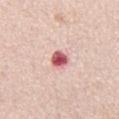Impression: Imaged during a routine full-body skin examination; the lesion was not biopsied and no histopathology is available. Acquisition and patient details: A region of skin cropped from a whole-body photographic capture, roughly 15 mm wide. Longest diameter approximately 2.5 mm. An algorithmic analysis of the crop reported a lesion color around L≈59 a*≈32 b*≈22 in CIELAB, about 19 CIELAB-L* units darker than the surrounding skin, and a normalized border contrast of about 11.5. The analysis additionally found a border-irregularity index near 2/10 and internal color variation of about 6 on a 0–10 scale. The analysis additionally found an automated nevus-likeness rating near 0 out of 100 and lesion-presence confidence of about 100/100. Imaged with white-light lighting. The subject is a male approximately 50 years of age. Located on the chest.